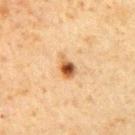workup=catalogued during a skin exam; not biopsied
automated lesion analysis=roughly 13 lightness units darker than nearby skin and a normalized border contrast of about 9.5; a border-irregularity rating of about 3/10 and a color-variation rating of about 9/10
tile lighting=cross-polarized illumination
anatomic site=the right upper arm
subject=male, aged 58 to 62
imaging modality=total-body-photography crop, ~15 mm field of view